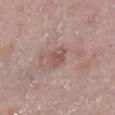Q: Was this lesion biopsied?
A: imaged on a skin check; not biopsied
Q: Patient demographics?
A: female, roughly 55 years of age
Q: Lesion location?
A: the left lower leg
Q: What kind of image is this?
A: total-body-photography crop, ~15 mm field of view